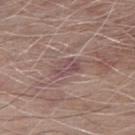Imaged during a routine full-body skin examination; the lesion was not biopsied and no histopathology is available. A male subject, about 65 years old. The lesion's longest dimension is about 3 mm. Imaged with white-light lighting. A 15 mm crop from a total-body photograph taken for skin-cancer surveillance. From the left forearm.